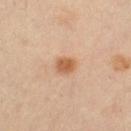Q: Was a biopsy performed?
A: total-body-photography surveillance lesion; no biopsy